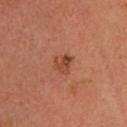workup=no biopsy performed (imaged during a skin exam).